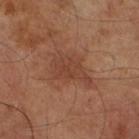This lesion was catalogued during total-body skin photography and was not selected for biopsy. The lesion is located on the right lower leg. A close-up tile cropped from a whole-body skin photograph, about 15 mm across. The patient is a male aged around 70.